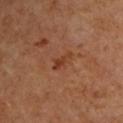biopsy status = imaged on a skin check; not biopsied
image = ~15 mm tile from a whole-body skin photo
lesion size = ~3 mm (longest diameter)
tile lighting = cross-polarized illumination
subject = male, roughly 60 years of age
anatomic site = the upper back